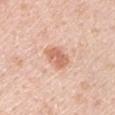notes = catalogued during a skin exam; not biopsied